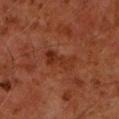Part of a total-body skin-imaging series; this lesion was reviewed on a skin check and was not flagged for biopsy. Captured under cross-polarized illumination. The recorded lesion diameter is about 4.5 mm. A male patient, aged 58 to 62. From the arm. A 15 mm crop from a total-body photograph taken for skin-cancer surveillance.